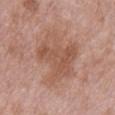Q: Is there a histopathology result?
A: imaged on a skin check; not biopsied